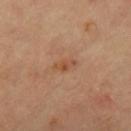Assessment: The lesion was photographed on a routine skin check and not biopsied; there is no pathology result. Background: A female subject roughly 60 years of age. The recorded lesion diameter is about 2.5 mm. This image is a 15 mm lesion crop taken from a total-body photograph. The lesion is on the left leg. Automated image analysis of the tile measured a footprint of about 2 mm², an eccentricity of roughly 0.95, and two-axis asymmetry of about 0.6. And it measured a lesion color around L≈50 a*≈23 b*≈34 in CIELAB, about 8 CIELAB-L* units darker than the surrounding skin, and a normalized border contrast of about 6.5. The analysis additionally found a within-lesion color-variation index near 0/10 and peripheral color asymmetry of about 0. The software also gave a nevus-likeness score of about 0/100 and lesion-presence confidence of about 100/100. The tile uses cross-polarized illumination.